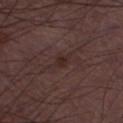<record>
<biopsy_status>not biopsied; imaged during a skin examination</biopsy_status>
<lesion_size>
  <long_diameter_mm_approx>3.0</long_diameter_mm_approx>
</lesion_size>
<patient>
  <sex>male</sex>
  <age_approx>50</age_approx>
</patient>
<automated_metrics>
  <area_mm2_approx>3.5</area_mm2_approx>
  <eccentricity>0.9</eccentricity>
  <shape_asymmetry>0.3</shape_asymmetry>
  <cielab_L>27</cielab_L>
  <cielab_a>17</cielab_a>
  <cielab_b>18</cielab_b>
  <vs_skin_contrast_norm>6.5</vs_skin_contrast_norm>
</automated_metrics>
<lighting>white-light</lighting>
<image>
  <source>total-body photography crop</source>
  <field_of_view_mm>15</field_of_view_mm>
</image>
<site>left thigh</site>
</record>